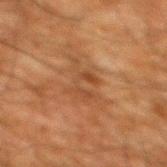The lesion was tiled from a total-body skin photograph and was not biopsied. The lesion is located on the mid back. This is a cross-polarized tile. A male subject, aged approximately 60. A roughly 15 mm field-of-view crop from a total-body skin photograph. About 4 mm across.